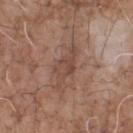{
  "biopsy_status": "not biopsied; imaged during a skin examination",
  "site": "chest",
  "lighting": "white-light",
  "image": {
    "source": "total-body photography crop",
    "field_of_view_mm": 15
  },
  "lesion_size": {
    "long_diameter_mm_approx": 5.5
  },
  "patient": {
    "sex": "male",
    "age_approx": 70
  },
  "automated_metrics": {
    "cielab_L": 46,
    "cielab_a": 19,
    "cielab_b": 25,
    "vs_skin_darker_L": 8.0
  }
}